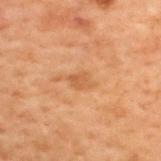biopsy status — total-body-photography surveillance lesion; no biopsy
illumination — cross-polarized
image — ~15 mm tile from a whole-body skin photo
site — the upper back
patient — male, approximately 70 years of age
image-analysis metrics — a symmetry-axis asymmetry near 0.3; a classifier nevus-likeness of about 0/100